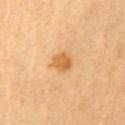Q: Was a biopsy performed?
A: total-body-photography surveillance lesion; no biopsy
Q: What are the patient's age and sex?
A: male, aged around 85 or older
Q: What is the imaging modality?
A: total-body-photography crop, ~15 mm field of view
Q: What is the anatomic site?
A: the left upper arm
Q: Automated lesion metrics?
A: a border-irregularity rating of about 2/10, a within-lesion color-variation index near 3/10, and radial color variation of about 1